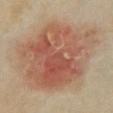biopsy_status: not biopsied; imaged during a skin examination
automated_metrics:
  vs_skin_darker_L: 10.0
  vs_skin_contrast_norm: 7.5
image:
  source: total-body photography crop
  field_of_view_mm: 15
patient:
  sex: female
  age_approx: 35
site: chest
lighting: cross-polarized